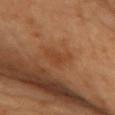Notes:
• workup · total-body-photography surveillance lesion; no biopsy
• lesion size · about 3.5 mm
• patient · female, in their 60s
• location · the chest
• image source · ~15 mm tile from a whole-body skin photo